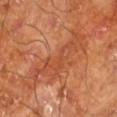A male patient, aged approximately 65.
Cropped from a whole-body photographic skin survey; the tile spans about 15 mm.
About 8 mm across.
Located on the left lower leg.